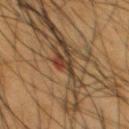No biopsy was performed on this lesion — it was imaged during a full skin examination and was not determined to be concerning. Located on the chest. A male subject, in their mid-50s. A lesion tile, about 15 mm wide, cut from a 3D total-body photograph. This is a cross-polarized tile. Longest diameter approximately 2.5 mm.